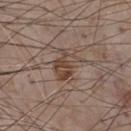Impression: Recorded during total-body skin imaging; not selected for excision or biopsy. Context: About 3.5 mm across. A male subject, approximately 75 years of age. This image is a 15 mm lesion crop taken from a total-body photograph. On the chest.